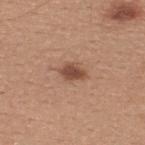Case summary:
– notes: imaged on a skin check; not biopsied
– lighting: white-light
– patient: male, roughly 30 years of age
– automated metrics: a mean CIELAB color near L≈49 a*≈21 b*≈29, about 12 CIELAB-L* units darker than the surrounding skin, and a lesion-to-skin contrast of about 8.5 (normalized; higher = more distinct)
– imaging modality: total-body-photography crop, ~15 mm field of view
– lesion size: ≈3 mm
– body site: the upper back